An algorithmic analysis of the crop reported a lesion area of about 18 mm², an outline eccentricity of about 0.85 (0 = round, 1 = elongated), and a shape-asymmetry score of about 0.2 (0 = symmetric). The software also gave an automated nevus-likeness rating near 85 out of 100 · the tile uses white-light illumination · the lesion is located on the back · the lesion's longest dimension is about 6.5 mm · a male patient, about 40 years old · a lesion tile, about 15 mm wide, cut from a 3D total-body photograph:
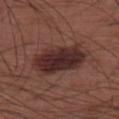The lesion was biopsied, and histopathology showed a melanoma in situ.